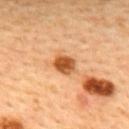The subject is a male about 45 years old.
On the upper back.
The tile uses cross-polarized illumination.
Longest diameter approximately 3 mm.
This image is a 15 mm lesion crop taken from a total-body photograph.